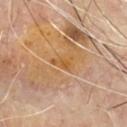biopsy status: imaged on a skin check; not biopsied
illumination: cross-polarized
size: ≈2.5 mm
subject: male, aged 63–67
site: the front of the torso
imaging modality: ~15 mm crop, total-body skin-cancer survey